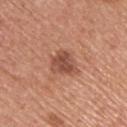Part of a total-body skin-imaging series; this lesion was reviewed on a skin check and was not flagged for biopsy.
The total-body-photography lesion software estimated an area of roughly 6.5 mm², an eccentricity of roughly 0.5, and a shape-asymmetry score of about 0.4 (0 = symmetric). The analysis additionally found a mean CIELAB color near L≈49 a*≈25 b*≈30, about 11 CIELAB-L* units darker than the surrounding skin, and a lesion-to-skin contrast of about 8 (normalized; higher = more distinct).
This is a white-light tile.
The patient is a female aged around 65.
From the left upper arm.
A close-up tile cropped from a whole-body skin photograph, about 15 mm across.
Approximately 3.5 mm at its widest.